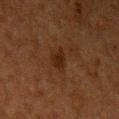Case summary:
- workup — catalogued during a skin exam; not biopsied
- automated lesion analysis — a footprint of about 3.5 mm² and a shape eccentricity near 0.85; border irregularity of about 3 on a 0–10 scale, a within-lesion color-variation index near 1.5/10, and peripheral color asymmetry of about 0.5; a classifier nevus-likeness of about 10/100 and a lesion-detection confidence of about 100/100
- acquisition — 15 mm crop, total-body photography
- lighting — cross-polarized illumination
- lesion diameter — ≈3 mm
- body site — the left upper arm
- subject — male, approximately 60 years of age